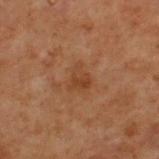Assessment: No biopsy was performed on this lesion — it was imaged during a full skin examination and was not determined to be concerning. Context: A 15 mm crop from a total-body photograph taken for skin-cancer surveillance. The tile uses cross-polarized illumination. The subject is a male approximately 70 years of age. The lesion is located on the upper back.